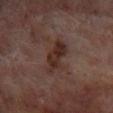No biopsy was performed on this lesion — it was imaged during a full skin examination and was not determined to be concerning. The subject is a male in their 70s. Cropped from a whole-body photographic skin survey; the tile spans about 15 mm. Automated image analysis of the tile measured a footprint of about 9 mm² and an outline eccentricity of about 0.95 (0 = round, 1 = elongated). The analysis additionally found an average lesion color of about L≈28 a*≈17 b*≈21 (CIELAB) and about 9 CIELAB-L* units darker than the surrounding skin. The software also gave a border-irregularity rating of about 4/10, a within-lesion color-variation index near 3.5/10, and peripheral color asymmetry of about 1. And it measured a nevus-likeness score of about 0/100 and lesion-presence confidence of about 100/100. Captured under cross-polarized illumination. Located on the left lower leg. The recorded lesion diameter is about 5.5 mm.